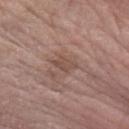notes: imaged on a skin check; not biopsied
subject: female, aged around 60
lesion size: ≈3 mm
site: the arm
image: ~15 mm crop, total-body skin-cancer survey
TBP lesion metrics: a classifier nevus-likeness of about 0/100 and lesion-presence confidence of about 95/100
illumination: white-light illumination